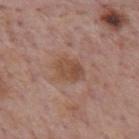{
  "biopsy_status": "not biopsied; imaged during a skin examination",
  "automated_metrics": {
    "color_variation_0_10": 3.5,
    "peripheral_color_asymmetry": 1.0
  },
  "patient": {
    "sex": "male",
    "age_approx": 75
  },
  "image": {
    "source": "total-body photography crop",
    "field_of_view_mm": 15
  },
  "lighting": "white-light",
  "site": "mid back",
  "lesion_size": {
    "long_diameter_mm_approx": 3.5
  }
}